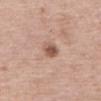The lesion was photographed on a routine skin check and not biopsied; there is no pathology result. Approximately 3 mm at its widest. The tile uses white-light illumination. A female subject aged 63 to 67. Cropped from a total-body skin-imaging series; the visible field is about 15 mm. From the upper back.